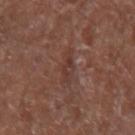notes: imaged on a skin check; not biopsied | subject: female, roughly 80 years of age | anatomic site: the right forearm | acquisition: ~15 mm crop, total-body skin-cancer survey.